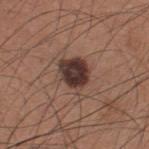This lesion was catalogued during total-body skin photography and was not selected for biopsy. The tile uses white-light illumination. Automated image analysis of the tile measured an average lesion color of about L≈36 a*≈17 b*≈21 (CIELAB) and a lesion-to-skin contrast of about 12.5 (normalized; higher = more distinct). The software also gave a nevus-likeness score of about 55/100 and lesion-presence confidence of about 100/100. About 4.5 mm across. The lesion is on the upper back. A male patient roughly 35 years of age. Cropped from a total-body skin-imaging series; the visible field is about 15 mm.